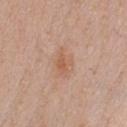Captured during whole-body skin photography for melanoma surveillance; the lesion was not biopsied. On the chest. Cropped from a whole-body photographic skin survey; the tile spans about 15 mm. The lesion's longest dimension is about 3.5 mm. Automated tile analysis of the lesion measured a shape eccentricity near 0.75 and a shape-asymmetry score of about 0.3 (0 = symmetric). It also reported internal color variation of about 2.5 on a 0–10 scale and a peripheral color-asymmetry measure near 0.5. Captured under white-light illumination. A male subject aged 38–42.